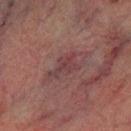biopsy_status: not biopsied; imaged during a skin examination
lighting: cross-polarized
site: leg
image:
  source: total-body photography crop
  field_of_view_mm: 15
patient:
  sex: male
  age_approx: 75